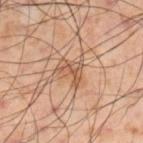{"biopsy_status": "not biopsied; imaged during a skin examination", "lighting": "cross-polarized", "lesion_size": {"long_diameter_mm_approx": 3.5}, "site": "right lower leg", "image": {"source": "total-body photography crop", "field_of_view_mm": 15}, "patient": {"sex": "male", "age_approx": 55}, "automated_metrics": {"vs_skin_darker_L": 9.0, "nevus_likeness_0_100": 40, "lesion_detection_confidence_0_100": 100}}